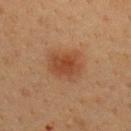Impression: The lesion was tiled from a total-body skin photograph and was not biopsied. Image and clinical context: A close-up tile cropped from a whole-body skin photograph, about 15 mm across. The subject is a female aged approximately 40. Automated tile analysis of the lesion measured border irregularity of about 2 on a 0–10 scale, a within-lesion color-variation index near 3/10, and a peripheral color-asymmetry measure near 1. And it measured a classifier nevus-likeness of about 100/100 and a lesion-detection confidence of about 100/100. On the upper back.